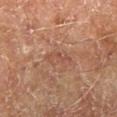Q: Was this lesion biopsied?
A: imaged on a skin check; not biopsied
Q: What is the anatomic site?
A: the right lower leg
Q: Lesion size?
A: ~4 mm (longest diameter)
Q: Who is the patient?
A: male, approximately 70 years of age
Q: What lighting was used for the tile?
A: cross-polarized
Q: What is the imaging modality?
A: ~15 mm tile from a whole-body skin photo